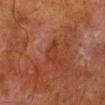biopsy status: no biopsy performed (imaged during a skin exam)
site: the leg
automated lesion analysis: a lesion area of about 4 mm², an eccentricity of roughly 0.75, and a shape-asymmetry score of about 0.35 (0 = symmetric); a border-irregularity index near 3/10; a nevus-likeness score of about 0/100 and lesion-presence confidence of about 100/100
subject: male, aged approximately 80
image: ~15 mm crop, total-body skin-cancer survey